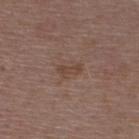The lesion was photographed on a routine skin check and not biopsied; there is no pathology result.
The tile uses white-light illumination.
From the upper back.
A female subject in their mid- to late 40s.
A roughly 15 mm field-of-view crop from a total-body skin photograph.
The lesion's longest dimension is about 2.5 mm.
Automated tile analysis of the lesion measured a lesion–skin lightness drop of about 6 and a lesion-to-skin contrast of about 6 (normalized; higher = more distinct). The software also gave a classifier nevus-likeness of about 0/100 and a detector confidence of about 100 out of 100 that the crop contains a lesion.